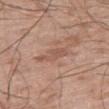notes = no biopsy performed (imaged during a skin exam)
anatomic site = the right thigh
acquisition = 15 mm crop, total-body photography
subject = male, about 60 years old
lighting = white-light illumination
lesion size = ~4 mm (longest diameter)